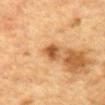* notes: catalogued during a skin exam; not biopsied
* anatomic site: the mid back
* patient: male, about 85 years old or older
* imaging modality: ~15 mm tile from a whole-body skin photo
* illumination: cross-polarized
* lesion size: about 3 mm